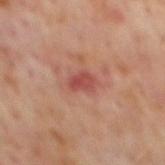Q: Was a biopsy performed?
A: imaged on a skin check; not biopsied
Q: Who is the patient?
A: male, in their mid-60s
Q: How was the tile lit?
A: cross-polarized
Q: Automated lesion metrics?
A: a border-irregularity rating of about 2.5/10, a color-variation rating of about 2.5/10, and a peripheral color-asymmetry measure near 1; an automated nevus-likeness rating near 0 out of 100 and a lesion-detection confidence of about 100/100
Q: Where on the body is the lesion?
A: the mid back
Q: How large is the lesion?
A: ~2.5 mm (longest diameter)
Q: How was this image acquired?
A: total-body-photography crop, ~15 mm field of view